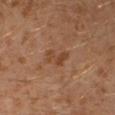Imaged during a routine full-body skin examination; the lesion was not biopsied and no histopathology is available.
Cropped from a whole-body photographic skin survey; the tile spans about 15 mm.
An algorithmic analysis of the crop reported a lesion–skin lightness drop of about 7 and a normalized lesion–skin contrast near 6.5. And it measured a border-irregularity index near 6.5/10, a within-lesion color-variation index near 0/10, and peripheral color asymmetry of about 0.
The tile uses cross-polarized illumination.
The subject is a male aged 28 to 32.
The recorded lesion diameter is about 3 mm.
From the leg.